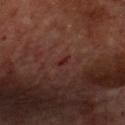workup: imaged on a skin check; not biopsied | subject: male, in their mid- to late 60s | automated lesion analysis: an average lesion color of about L≈24 a*≈28 b*≈21 (CIELAB), a lesion–skin lightness drop of about 6, and a normalized lesion–skin contrast near 7; a border-irregularity index near 2.5/10 and radial color variation of about 0; a nevus-likeness score of about 0/100 and a detector confidence of about 95 out of 100 that the crop contains a lesion | anatomic site: the front of the torso | illumination: cross-polarized | imaging modality: 15 mm crop, total-body photography | lesion diameter: about 1.5 mm.